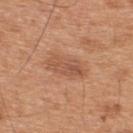A male patient, approximately 45 years of age. A close-up tile cropped from a whole-body skin photograph, about 15 mm across. About 4.5 mm across. This is a white-light tile. On the upper back.